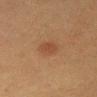Imaged during a routine full-body skin examination; the lesion was not biopsied and no histopathology is available.
The lesion-visualizer software estimated a footprint of about 4.5 mm² and an eccentricity of roughly 0.65. The software also gave a border-irregularity rating of about 1.5/10, a color-variation rating of about 1/10, and a peripheral color-asymmetry measure near 0.5. It also reported an automated nevus-likeness rating near 75 out of 100 and a lesion-detection confidence of about 100/100.
The tile uses cross-polarized illumination.
A close-up tile cropped from a whole-body skin photograph, about 15 mm across.
Located on the mid back.
Approximately 2.5 mm at its widest.
A female patient aged around 50.